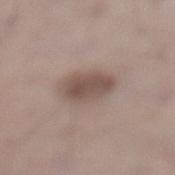Recorded during total-body skin imaging; not selected for excision or biopsy.
The lesion is located on the left lower leg.
The recorded lesion diameter is about 4.5 mm.
A 15 mm crop from a total-body photograph taken for skin-cancer surveillance.
The patient is a male aged 68 to 72.
This is a white-light tile.